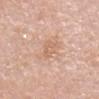| key | value |
|---|---|
| biopsy status | imaged on a skin check; not biopsied |
| patient | female, aged 58–62 |
| site | the head or neck |
| acquisition | 15 mm crop, total-body photography |